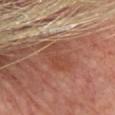Assessment: The lesion was photographed on a routine skin check and not biopsied; there is no pathology result. Image and clinical context: The lesion is on the front of the torso. This image is a 15 mm lesion crop taken from a total-body photograph. The lesion-visualizer software estimated a border-irregularity rating of about 3.5/10. The software also gave a detector confidence of about 100 out of 100 that the crop contains a lesion. The recorded lesion diameter is about 3.5 mm. Imaged with cross-polarized lighting. A female subject approximately 65 years of age.